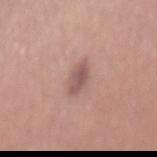Clinical impression: Part of a total-body skin-imaging series; this lesion was reviewed on a skin check and was not flagged for biopsy. Context: Measured at roughly 3.5 mm in maximum diameter. The tile uses white-light illumination. A roughly 15 mm field-of-view crop from a total-body skin photograph. An algorithmic analysis of the crop reported a border-irregularity rating of about 2.5/10, a within-lesion color-variation index near 3/10, and peripheral color asymmetry of about 1. The subject is a female aged approximately 50. The lesion is located on the mid back.